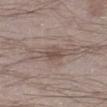Notes:
• workup: no biopsy performed (imaged during a skin exam)
• subject: male, aged 23–27
• acquisition: 15 mm crop, total-body photography
• location: the left lower leg
• image-analysis metrics: an area of roughly 5 mm², an eccentricity of roughly 0.85, and a symmetry-axis asymmetry near 0.25; an average lesion color of about L≈49 a*≈14 b*≈22 (CIELAB) and a lesion-to-skin contrast of about 7 (normalized; higher = more distinct); an automated nevus-likeness rating near 0 out of 100
• illumination: white-light